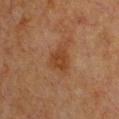Assessment:
This lesion was catalogued during total-body skin photography and was not selected for biopsy.
Image and clinical context:
Measured at roughly 3 mm in maximum diameter. The patient is a female aged around 55. Located on the chest. This is a cross-polarized tile. A 15 mm close-up extracted from a 3D total-body photography capture.